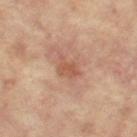Captured during whole-body skin photography for melanoma surveillance; the lesion was not biopsied.
A region of skin cropped from a whole-body photographic capture, roughly 15 mm wide.
A female subject about 65 years old.
On the right leg.